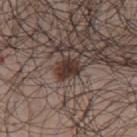Clinical impression:
No biopsy was performed on this lesion — it was imaged during a full skin examination and was not determined to be concerning.
Acquisition and patient details:
A male patient in their 50s. A lesion tile, about 15 mm wide, cut from a 3D total-body photograph. Located on the upper back. The recorded lesion diameter is about 2.5 mm. This is a white-light tile.